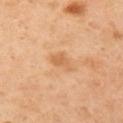<case>
  <biopsy_status>not biopsied; imaged during a skin examination</biopsy_status>
  <patient>
    <sex>female</sex>
    <age_approx>40</age_approx>
  </patient>
  <site>left upper arm</site>
  <automated_metrics>
    <area_mm2_approx>3.5</area_mm2_approx>
    <shape_asymmetry>0.35</shape_asymmetry>
  </automated_metrics>
  <image>
    <source>total-body photography crop</source>
    <field_of_view_mm>15</field_of_view_mm>
  </image>
  <lighting>cross-polarized</lighting>
  <lesion_size>
    <long_diameter_mm_approx>3.0</long_diameter_mm_approx>
  </lesion_size>
</case>